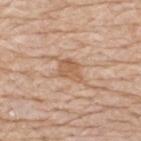Part of a total-body skin-imaging series; this lesion was reviewed on a skin check and was not flagged for biopsy. Captured under white-light illumination. The patient is a male about 80 years old. A 15 mm crop from a total-body photograph taken for skin-cancer surveillance. The lesion is on the upper back. About 4 mm across.